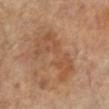{
  "biopsy_status": "not biopsied; imaged during a skin examination",
  "lesion_size": {
    "long_diameter_mm_approx": 7.0
  },
  "site": "right leg",
  "patient": {
    "sex": "female",
    "age_approx": 65
  },
  "image": {
    "source": "total-body photography crop",
    "field_of_view_mm": 15
  },
  "lighting": "cross-polarized"
}